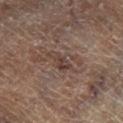<lesion>
<biopsy_status>not biopsied; imaged during a skin examination</biopsy_status>
<lighting>cross-polarized</lighting>
<patient>
  <sex>male</sex>
  <age_approx>65</age_approx>
</patient>
<site>right lower leg</site>
<image>
  <source>total-body photography crop</source>
  <field_of_view_mm>15</field_of_view_mm>
</image>
<lesion_size>
  <long_diameter_mm_approx>3.0</long_diameter_mm_approx>
</lesion_size>
</lesion>